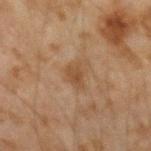Q: Was this lesion biopsied?
A: total-body-photography surveillance lesion; no biopsy
Q: Lesion size?
A: about 2.5 mm
Q: Who is the patient?
A: male, in their mid-40s
Q: Lesion location?
A: the left forearm
Q: What lighting was used for the tile?
A: cross-polarized illumination
Q: What is the imaging modality?
A: total-body-photography crop, ~15 mm field of view
Q: Automated lesion metrics?
A: a footprint of about 4 mm², an outline eccentricity of about 0.65 (0 = round, 1 = elongated), and a symmetry-axis asymmetry near 0.35; a mean CIELAB color near L≈38 a*≈16 b*≈28 and a lesion-to-skin contrast of about 6.5 (normalized; higher = more distinct)